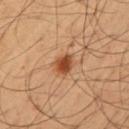Assessment: Part of a total-body skin-imaging series; this lesion was reviewed on a skin check and was not flagged for biopsy. Clinical summary: A male subject approximately 55 years of age. A 15 mm crop from a total-body photograph taken for skin-cancer surveillance. On the left upper arm.